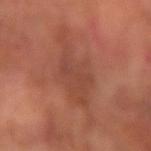Impression:
Part of a total-body skin-imaging series; this lesion was reviewed on a skin check and was not flagged for biopsy.
Context:
Located on the left forearm. A region of skin cropped from a whole-body photographic capture, roughly 15 mm wide. An algorithmic analysis of the crop reported a footprint of about 14 mm², an outline eccentricity of about 0.75 (0 = round, 1 = elongated), and two-axis asymmetry of about 0.35. And it measured lesion-presence confidence of about 100/100. Approximately 6.5 mm at its widest. A male subject approximately 65 years of age.